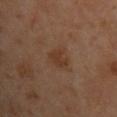This lesion was catalogued during total-body skin photography and was not selected for biopsy. This is a cross-polarized tile. The lesion's longest dimension is about 2.5 mm. A lesion tile, about 15 mm wide, cut from a 3D total-body photograph. A male patient approximately 40 years of age. The lesion is on the front of the torso.